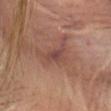Background: The total-body-photography lesion software estimated a footprint of about 4 mm². The analysis additionally found a nevus-likeness score of about 0/100 and lesion-presence confidence of about 80/100. The lesion is located on the head or neck. The patient is a female roughly 80 years of age. Cropped from a whole-body photographic skin survey; the tile spans about 15 mm. This is a cross-polarized tile.